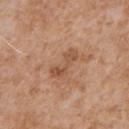Clinical impression:
Part of a total-body skin-imaging series; this lesion was reviewed on a skin check and was not flagged for biopsy.
Clinical summary:
The lesion is located on the front of the torso. A male subject, about 65 years old. Cropped from a total-body skin-imaging series; the visible field is about 15 mm.